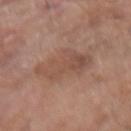<record>
  <lesion_size>
    <long_diameter_mm_approx>5.5</long_diameter_mm_approx>
  </lesion_size>
  <site>right upper arm</site>
  <lighting>white-light</lighting>
  <patient>
    <sex>male</sex>
    <age_approx>75</age_approx>
  </patient>
  <image>
    <source>total-body photography crop</source>
    <field_of_view_mm>15</field_of_view_mm>
  </image>
</record>